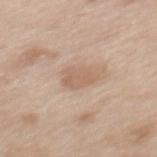notes: total-body-photography surveillance lesion; no biopsy
subject: female, roughly 40 years of age
image-analysis metrics: an average lesion color of about L≈61 a*≈17 b*≈29 (CIELAB), roughly 8 lightness units darker than nearby skin, and a lesion-to-skin contrast of about 5.5 (normalized; higher = more distinct)
lighting: white-light illumination
size: ≈3.5 mm
acquisition: 15 mm crop, total-body photography
body site: the mid back